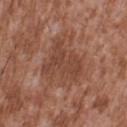follow-up: no biopsy performed (imaged during a skin exam)
acquisition: total-body-photography crop, ~15 mm field of view
subject: male, aged 43 to 47
automated metrics: a footprint of about 12 mm² and an eccentricity of roughly 0.7
location: the back